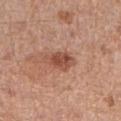Notes:
- workup · total-body-photography surveillance lesion; no biopsy
- patient · female, aged around 50
- diameter · ~3.5 mm (longest diameter)
- body site · the right forearm
- image-analysis metrics · an area of roughly 6.5 mm²; a lesion–skin lightness drop of about 11 and a normalized border contrast of about 7.5; a classifier nevus-likeness of about 65/100 and lesion-presence confidence of about 100/100
- image · total-body-photography crop, ~15 mm field of view
- illumination · white-light illumination